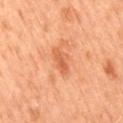biopsy status = imaged on a skin check; not biopsied | image = ~15 mm crop, total-body skin-cancer survey | illumination = cross-polarized | image-analysis metrics = a color-variation rating of about 2.5/10 and peripheral color asymmetry of about 0.5 | patient = male, aged around 60 | lesion size = ≈3.5 mm | body site = the abdomen.